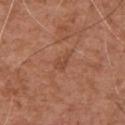Imaged during a routine full-body skin examination; the lesion was not biopsied and no histopathology is available. The total-body-photography lesion software estimated an eccentricity of roughly 0.8 and a symmetry-axis asymmetry near 0.45. The software also gave about 6 CIELAB-L* units darker than the surrounding skin. It also reported border irregularity of about 4.5 on a 0–10 scale, internal color variation of about 0 on a 0–10 scale, and radial color variation of about 0. And it measured a classifier nevus-likeness of about 0/100 and lesion-presence confidence of about 100/100. A male subject aged approximately 75. Captured under white-light illumination. About 2.5 mm across. Located on the chest. A lesion tile, about 15 mm wide, cut from a 3D total-body photograph.